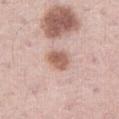No biopsy was performed on this lesion — it was imaged during a full skin examination and was not determined to be concerning.